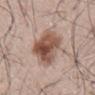| field | value |
|---|---|
| follow-up | imaged on a skin check; not biopsied |
| image-analysis metrics | an average lesion color of about L≈52 a*≈19 b*≈26 (CIELAB), about 14 CIELAB-L* units darker than the surrounding skin, and a normalized border contrast of about 10; a border-irregularity rating of about 3/10, internal color variation of about 7 on a 0–10 scale, and radial color variation of about 2 |
| body site | the mid back |
| size | about 8 mm |
| imaging modality | total-body-photography crop, ~15 mm field of view |
| subject | male, in their mid-60s |
| illumination | white-light |